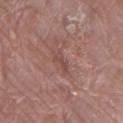Assessment: The lesion was tiled from a total-body skin photograph and was not biopsied. Clinical summary: On the left thigh. Longest diameter approximately 2.5 mm. This is a white-light tile. A roughly 15 mm field-of-view crop from a total-body skin photograph. The patient is a female in their 70s.